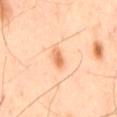<tbp_lesion>
<biopsy_status>not biopsied; imaged during a skin examination</biopsy_status>
<image>
  <source>total-body photography crop</source>
  <field_of_view_mm>15</field_of_view_mm>
</image>
<patient>
  <sex>male</sex>
  <age_approx>45</age_approx>
</patient>
<site>back</site>
<lighting>cross-polarized</lighting>
</tbp_lesion>